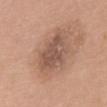<record>
  <biopsy_status>not biopsied; imaged during a skin examination</biopsy_status>
  <automated_metrics>
    <area_mm2_approx>15.0</area_mm2_approx>
    <shape_asymmetry>0.25</shape_asymmetry>
    <border_irregularity_0_10>3.0</border_irregularity_0_10>
    <color_variation_0_10>4.0</color_variation_0_10>
    <peripheral_color_asymmetry>1.5</peripheral_color_asymmetry>
  </automated_metrics>
  <site>upper back</site>
  <lesion_size>
    <long_diameter_mm_approx>6.0</long_diameter_mm_approx>
  </lesion_size>
  <image>
    <source>total-body photography crop</source>
    <field_of_view_mm>15</field_of_view_mm>
  </image>
  <patient>
    <sex>female</sex>
    <age_approx>60</age_approx>
  </patient>
</record>